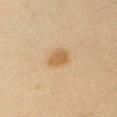Impression: Imaged during a routine full-body skin examination; the lesion was not biopsied and no histopathology is available. Background: The lesion is located on the right upper arm. Longest diameter approximately 3 mm. A close-up tile cropped from a whole-body skin photograph, about 15 mm across. A male patient about 45 years old. Imaged with cross-polarized lighting.